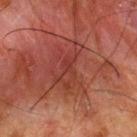The lesion is located on the upper back.
A male patient roughly 65 years of age.
A region of skin cropped from a whole-body photographic capture, roughly 15 mm wide.
Automated image analysis of the tile measured a mean CIELAB color near L≈30 a*≈25 b*≈23 and a lesion–skin lightness drop of about 5. And it measured a border-irregularity index near 8.5/10, a within-lesion color-variation index near 3.5/10, and peripheral color asymmetry of about 1.
Approximately 5.5 mm at its widest.